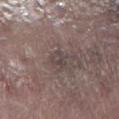Notes:
– notes · catalogued during a skin exam; not biopsied
– subject · male, roughly 75 years of age
– site · the right lower leg
– TBP lesion metrics · an area of roughly 3 mm² and a symmetry-axis asymmetry near 0.45; a lesion color around L≈42 a*≈12 b*≈16 in CIELAB and a lesion-to-skin contrast of about 5.5 (normalized; higher = more distinct); a border-irregularity index near 4/10, a within-lesion color-variation index near 3.5/10, and peripheral color asymmetry of about 1.5
– acquisition · ~15 mm tile from a whole-body skin photo
– lesion size · ≈2.5 mm